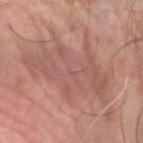Imaged during a routine full-body skin examination; the lesion was not biopsied and no histopathology is available. The lesion is located on the left forearm. Longest diameter approximately 11 mm. A region of skin cropped from a whole-body photographic capture, roughly 15 mm wide. A male subject aged approximately 65.